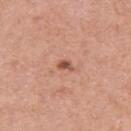Clinical impression:
The lesion was tiled from a total-body skin photograph and was not biopsied.
Background:
The patient is a female in their 40s. The recorded lesion diameter is about 2 mm. A lesion tile, about 15 mm wide, cut from a 3D total-body photograph. The lesion-visualizer software estimated an area of roughly 2 mm². Captured under white-light illumination. From the left upper arm.